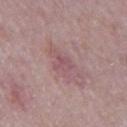  lighting: white-light
  lesion_size:
    long_diameter_mm_approx: 2.5
  site: mid back
  image:
    source: total-body photography crop
    field_of_view_mm: 15
  automated_metrics:
    area_mm2_approx: 3.5
    eccentricity: 0.7
    shape_asymmetry: 0.35
    cielab_L: 52
    cielab_a: 23
    cielab_b: 16
    vs_skin_darker_L: 6.0
    vs_skin_contrast_norm: 5.0
    border_irregularity_0_10: 3.5
    color_variation_0_10: 2.5
    peripheral_color_asymmetry: 1.0
  patient:
    sex: male
    age_approx: 65